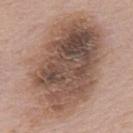This lesion was catalogued during total-body skin photography and was not selected for biopsy.
A roughly 15 mm field-of-view crop from a total-body skin photograph.
Measured at roughly 14 mm in maximum diameter.
Automated image analysis of the tile measured an area of roughly 85 mm² and two-axis asymmetry of about 0.15.
Located on the upper back.
A male subject, in their mid- to late 50s.